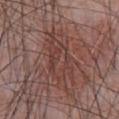<record>
  <biopsy_status>not biopsied; imaged during a skin examination</biopsy_status>
  <patient>
    <sex>male</sex>
    <age_approx>65</age_approx>
  </patient>
  <lighting>white-light</lighting>
  <site>abdomen</site>
  <image>
    <source>total-body photography crop</source>
    <field_of_view_mm>15</field_of_view_mm>
  </image>
  <automated_metrics>
    <shape_asymmetry>0.4</shape_asymmetry>
    <cielab_L>40</cielab_L>
    <cielab_a>20</cielab_a>
    <cielab_b>22</cielab_b>
    <vs_skin_darker_L>6.0</vs_skin_darker_L>
    <border_irregularity_0_10>5.0</border_irregularity_0_10>
    <color_variation_0_10>4.5</color_variation_0_10>
    <peripheral_color_asymmetry>1.5</peripheral_color_asymmetry>
  </automated_metrics>
</record>